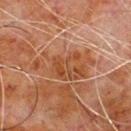Imaged during a routine full-body skin examination; the lesion was not biopsied and no histopathology is available.
From the chest.
A male subject, roughly 80 years of age.
The lesion-visualizer software estimated a shape eccentricity near 0.9 and two-axis asymmetry of about 0.35. The analysis additionally found a mean CIELAB color near L≈38 a*≈20 b*≈29. It also reported a border-irregularity index near 5.5/10, internal color variation of about 5 on a 0–10 scale, and a peripheral color-asymmetry measure near 1.5. It also reported a classifier nevus-likeness of about 0/100.
About 7.5 mm across.
A 15 mm close-up extracted from a 3D total-body photography capture.